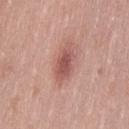Notes:
- workup — no biopsy performed (imaged during a skin exam)
- size — ≈4.5 mm
- patient — female, aged 38–42
- lighting — white-light illumination
- imaging modality — 15 mm crop, total-body photography
- site — the lower back
- TBP lesion metrics — a lesion color around L≈54 a*≈25 b*≈24 in CIELAB, a lesion–skin lightness drop of about 12, and a lesion-to-skin contrast of about 8 (normalized; higher = more distinct); a border-irregularity rating of about 3/10, internal color variation of about 2.5 on a 0–10 scale, and radial color variation of about 1; a detector confidence of about 100 out of 100 that the crop contains a lesion